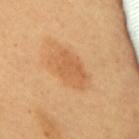| feature | finding |
|---|---|
| workup | imaged on a skin check; not biopsied |
| site | the upper back |
| illumination | cross-polarized illumination |
| imaging modality | total-body-photography crop, ~15 mm field of view |
| lesion diameter | ≈5.5 mm |
| patient | female, aged 58–62 |